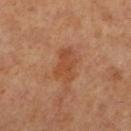image = ~15 mm crop, total-body skin-cancer survey | patient = female, in their mid- to late 50s | TBP lesion metrics = a footprint of about 8 mm² and a symmetry-axis asymmetry near 0.35; a border-irregularity index near 4/10, internal color variation of about 3 on a 0–10 scale, and radial color variation of about 1 | site = the left lower leg.